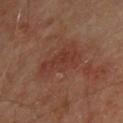Clinical summary: The patient is a male aged approximately 65. On the upper back. The lesion's longest dimension is about 5 mm. Cropped from a total-body skin-imaging series; the visible field is about 15 mm.